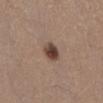Automated tile analysis of the lesion measured a lesion area of about 5.5 mm², an outline eccentricity of about 0.55 (0 = round, 1 = elongated), and a symmetry-axis asymmetry near 0.15. The analysis additionally found a lesion–skin lightness drop of about 14 and a normalized lesion–skin contrast near 10.5. And it measured a border-irregularity index near 1.5/10 and peripheral color asymmetry of about 1.5. The analysis additionally found a detector confidence of about 100 out of 100 that the crop contains a lesion.
Measured at roughly 3 mm in maximum diameter.
From the lower back.
Cropped from a whole-body photographic skin survey; the tile spans about 15 mm.
A male patient, about 55 years old.